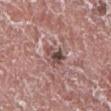| feature | finding |
|---|---|
| follow-up | total-body-photography surveillance lesion; no biopsy |
| image | ~15 mm crop, total-body skin-cancer survey |
| TBP lesion metrics | a border-irregularity index near 4.5/10, a within-lesion color-variation index near 4.5/10, and a peripheral color-asymmetry measure near 2; a nevus-likeness score of about 0/100 and lesion-presence confidence of about 95/100 |
| patient | male, aged 73–77 |
| anatomic site | the left lower leg |
| illumination | white-light |
| lesion size | about 3 mm |